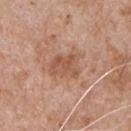| feature | finding |
|---|---|
| notes | no biopsy performed (imaged during a skin exam) |
| image source | ~15 mm crop, total-body skin-cancer survey |
| subject | male, about 65 years old |
| anatomic site | the front of the torso |
| TBP lesion metrics | a border-irregularity rating of about 3/10, internal color variation of about 3 on a 0–10 scale, and a peripheral color-asymmetry measure near 1; a classifier nevus-likeness of about 0/100 and a detector confidence of about 100 out of 100 that the crop contains a lesion |